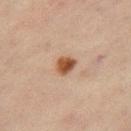Assessment:
The lesion was tiled from a total-body skin photograph and was not biopsied.
Image and clinical context:
On the right thigh. An algorithmic analysis of the crop reported an area of roughly 4.5 mm², an outline eccentricity of about 0.5 (0 = round, 1 = elongated), and a symmetry-axis asymmetry near 0.2. And it measured a mean CIELAB color near L≈45 a*≈20 b*≈30, about 13 CIELAB-L* units darker than the surrounding skin, and a lesion-to-skin contrast of about 11 (normalized; higher = more distinct). And it measured border irregularity of about 2 on a 0–10 scale, a color-variation rating of about 4/10, and peripheral color asymmetry of about 1.5. It also reported a detector confidence of about 100 out of 100 that the crop contains a lesion. Imaged with cross-polarized lighting. A region of skin cropped from a whole-body photographic capture, roughly 15 mm wide. The lesion's longest dimension is about 2.5 mm. A female subject, in their mid- to late 40s.